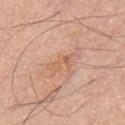{"biopsy_status": "not biopsied; imaged during a skin examination", "image": {"source": "total-body photography crop", "field_of_view_mm": 15}, "patient": {"sex": "male", "age_approx": 45}, "lighting": "white-light", "site": "chest"}